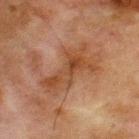notes = no biopsy performed (imaged during a skin exam)
site = the chest
imaging modality = ~15 mm tile from a whole-body skin photo
size = about 6.5 mm
subject = male, in their mid- to late 60s